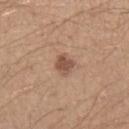Image and clinical context:
The lesion is located on the arm. A male subject, aged 43–47. Longest diameter approximately 2.5 mm. This image is a 15 mm lesion crop taken from a total-body photograph.